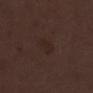| key | value |
|---|---|
| biopsy status | catalogued during a skin exam; not biopsied |
| imaging modality | ~15 mm crop, total-body skin-cancer survey |
| site | the left lower leg |
| patient | female, about 50 years old |
| illumination | white-light |